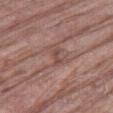Assessment:
The lesion was tiled from a total-body skin photograph and was not biopsied.
Acquisition and patient details:
This is a white-light tile. The lesion's longest dimension is about 2.5 mm. A male subject, roughly 80 years of age. From the right thigh. A lesion tile, about 15 mm wide, cut from a 3D total-body photograph.